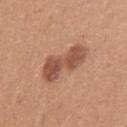Findings:
• notes — total-body-photography surveillance lesion; no biopsy
• patient — female, aged 38–42
• site — the left upper arm
• diameter — about 5.5 mm
• acquisition — ~15 mm tile from a whole-body skin photo